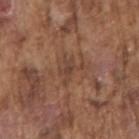Notes:
* biopsy status: no biopsy performed (imaged during a skin exam)
* site: the right upper arm
* image source: ~15 mm crop, total-body skin-cancer survey
* patient: male, aged approximately 75